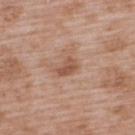<tbp_lesion>
<patient>
  <sex>male</sex>
  <age_approx>50</age_approx>
</patient>
<site>upper back</site>
<lesion_size>
  <long_diameter_mm_approx>2.5</long_diameter_mm_approx>
</lesion_size>
<lighting>white-light</lighting>
<automated_metrics>
  <border_irregularity_0_10>3.0</border_irregularity_0_10>
  <color_variation_0_10>2.0</color_variation_0_10>
  <peripheral_color_asymmetry>0.5</peripheral_color_asymmetry>
  <lesion_detection_confidence_0_100>100</lesion_detection_confidence_0_100>
</automated_metrics>
<image>
  <source>total-body photography crop</source>
  <field_of_view_mm>15</field_of_view_mm>
</image>
</tbp_lesion>